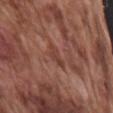| field | value |
|---|---|
| notes | imaged on a skin check; not biopsied |
| acquisition | ~15 mm crop, total-body skin-cancer survey |
| subject | male, approximately 75 years of age |
| size | about 3 mm |
| body site | the right upper arm |
| tile lighting | white-light illumination |